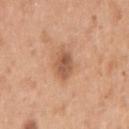Imaged during a routine full-body skin examination; the lesion was not biopsied and no histopathology is available. Captured under white-light illumination. About 3 mm across. A female subject, approximately 40 years of age. A region of skin cropped from a whole-body photographic capture, roughly 15 mm wide. On the right upper arm.